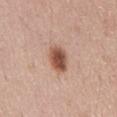• image source: 15 mm crop, total-body photography
• subject: male, aged approximately 65
• lighting: white-light illumination
• diameter: about 4 mm
• automated metrics: a footprint of about 7.5 mm², an eccentricity of roughly 0.8, and a shape-asymmetry score of about 0.15 (0 = symmetric); an average lesion color of about L≈53 a*≈22 b*≈29 (CIELAB) and about 15 CIELAB-L* units darker than the surrounding skin; a border-irregularity rating of about 1.5/10, a within-lesion color-variation index near 6/10, and peripheral color asymmetry of about 2; a classifier nevus-likeness of about 100/100 and a detector confidence of about 100 out of 100 that the crop contains a lesion
• body site: the abdomen